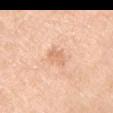Impression: This lesion was catalogued during total-body skin photography and was not selected for biopsy. Acquisition and patient details: Longest diameter approximately 3 mm. Cropped from a total-body skin-imaging series; the visible field is about 15 mm. A female patient aged 53–57. The lesion is on the left upper arm. Captured under white-light illumination.